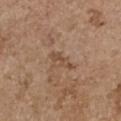follow-up = no biopsy performed (imaged during a skin exam); body site = the chest; illumination = white-light; patient = female, about 65 years old; image = total-body-photography crop, ~15 mm field of view; automated metrics = a nevus-likeness score of about 0/100 and a detector confidence of about 100 out of 100 that the crop contains a lesion; lesion size = ≈3 mm.